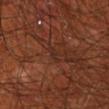<record>
<biopsy_status>not biopsied; imaged during a skin examination</biopsy_status>
<lesion_size>
  <long_diameter_mm_approx>3.0</long_diameter_mm_approx>
</lesion_size>
<site>right upper arm</site>
<patient>
  <sex>male</sex>
  <age_approx>70</age_approx>
</patient>
<image>
  <source>total-body photography crop</source>
  <field_of_view_mm>15</field_of_view_mm>
</image>
<lighting>cross-polarized</lighting>
</record>